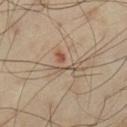tile lighting = cross-polarized
subject = male, aged approximately 55
anatomic site = the left thigh
acquisition = ~15 mm crop, total-body skin-cancer survey
lesion size = about 4 mm
automated lesion analysis = roughly 8 lightness units darker than nearby skin and a normalized lesion–skin contrast near 6; border irregularity of about 10 on a 0–10 scale, internal color variation of about 0 on a 0–10 scale, and radial color variation of about 0